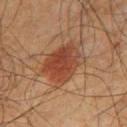| feature | finding |
|---|---|
| biopsy status | total-body-photography surveillance lesion; no biopsy |
| tile lighting | cross-polarized |
| size | about 5.5 mm |
| location | the left thigh |
| image source | ~15 mm crop, total-body skin-cancer survey |
| patient | male, aged around 60 |